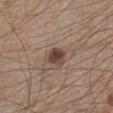From the left lower leg. The lesion's longest dimension is about 3 mm. Cropped from a total-body skin-imaging series; the visible field is about 15 mm. A male patient, roughly 45 years of age. The tile uses white-light illumination. The total-body-photography lesion software estimated an area of roughly 5.5 mm², an outline eccentricity of about 0.55 (0 = round, 1 = elongated), and two-axis asymmetry of about 0.25. The software also gave an average lesion color of about L≈43 a*≈16 b*≈24 (CIELAB), about 12 CIELAB-L* units darker than the surrounding skin, and a normalized border contrast of about 9.5.